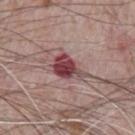This lesion was catalogued during total-body skin photography and was not selected for biopsy. The lesion is on the chest. Captured under white-light illumination. A male patient, aged 68 to 72. This image is a 15 mm lesion crop taken from a total-body photograph. About 4.5 mm across.